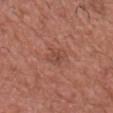follow-up: catalogued during a skin exam; not biopsied | patient: male, aged 73 to 77 | body site: the head or neck | illumination: white-light | acquisition: ~15 mm tile from a whole-body skin photo.